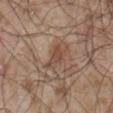Clinical impression: Captured during whole-body skin photography for melanoma surveillance; the lesion was not biopsied. Clinical summary: Automated image analysis of the tile measured an area of roughly 5 mm², an outline eccentricity of about 0.85 (0 = round, 1 = elongated), and a shape-asymmetry score of about 0.4 (0 = symmetric). And it measured a lesion color around L≈47 a*≈18 b*≈27 in CIELAB and a normalized lesion–skin contrast near 6.5. The software also gave border irregularity of about 4.5 on a 0–10 scale and a within-lesion color-variation index near 2/10. And it measured an automated nevus-likeness rating near 0 out of 100. A male subject, approximately 55 years of age. A 15 mm close-up tile from a total-body photography series done for melanoma screening. The recorded lesion diameter is about 3.5 mm. Captured under white-light illumination. The lesion is located on the front of the torso.